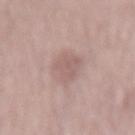  biopsy_status: not biopsied; imaged during a skin examination
  site: mid back
  automated_metrics:
    cielab_L: 60
    cielab_a: 18
    cielab_b: 21
    vs_skin_darker_L: 8.0
    border_irregularity_0_10: 2.5
    color_variation_0_10: 2.0
    peripheral_color_asymmetry: 0.5
  lighting: white-light
  lesion_size:
    long_diameter_mm_approx: 6.0
  image:
    source: total-body photography crop
    field_of_view_mm: 15
  patient:
    sex: male
    age_approx: 60